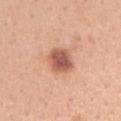Imaged during a routine full-body skin examination; the lesion was not biopsied and no histopathology is available. From the left upper arm. Longest diameter approximately 3.5 mm. Imaged with white-light lighting. The patient is a female aged 28–32. Automated image analysis of the tile measured a lesion area of about 8 mm², an eccentricity of roughly 0.55, and a symmetry-axis asymmetry near 0.2. This image is a 15 mm lesion crop taken from a total-body photograph.